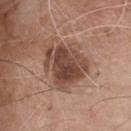  biopsy_status: not biopsied; imaged during a skin examination
  patient:
    sex: male
    age_approx: 80
  image:
    source: total-body photography crop
    field_of_view_mm: 15
  lighting: white-light
  site: chest
  automated_metrics:
    color_variation_0_10: 6.0
    peripheral_color_asymmetry: 2.0